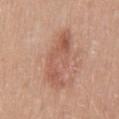follow-up — imaged on a skin check; not biopsied | acquisition — 15 mm crop, total-body photography | site — the mid back | subject — female, aged around 40.